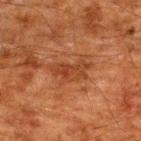The lesion's longest dimension is about 4.5 mm. A male patient, aged 58 to 62. The lesion is on the upper back. The tile uses cross-polarized illumination. A 15 mm crop from a total-body photograph taken for skin-cancer surveillance. Automated tile analysis of the lesion measured an area of roughly 7.5 mm², an outline eccentricity of about 0.85 (0 = round, 1 = elongated), and a symmetry-axis asymmetry near 0.35. The software also gave an automated nevus-likeness rating near 0 out of 100 and a detector confidence of about 100 out of 100 that the crop contains a lesion.